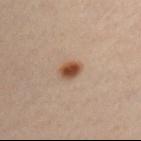Recorded during total-body skin imaging; not selected for excision or biopsy. Cropped from a whole-body photographic skin survey; the tile spans about 15 mm. A male subject, aged 33 to 37. This is a cross-polarized tile. Approximately 2.5 mm at its widest. An algorithmic analysis of the crop reported an area of roughly 4.5 mm². And it measured border irregularity of about 2 on a 0–10 scale, internal color variation of about 5 on a 0–10 scale, and radial color variation of about 1.5. The lesion is on the chest.